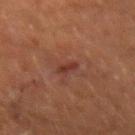follow-up — catalogued during a skin exam; not biopsied
subject — male, in their mid- to late 40s
anatomic site — the right forearm
tile lighting — cross-polarized illumination
TBP lesion metrics — a border-irregularity index near 3.5/10, a within-lesion color-variation index near 0/10, and radial color variation of about 0
acquisition — ~15 mm crop, total-body skin-cancer survey
size — about 2.5 mm